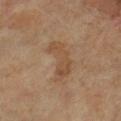Q: How was this image acquired?
A: ~15 mm tile from a whole-body skin photo
Q: Lesion size?
A: ≈5 mm
Q: Automated lesion metrics?
A: a footprint of about 9.5 mm² and two-axis asymmetry of about 0.5; a lesion color around L≈46 a*≈17 b*≈31 in CIELAB, a lesion–skin lightness drop of about 6, and a lesion-to-skin contrast of about 5.5 (normalized; higher = more distinct); an automated nevus-likeness rating near 0 out of 100
Q: Where on the body is the lesion?
A: the right lower leg
Q: What are the patient's age and sex?
A: female, aged 68 to 72